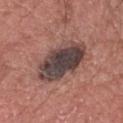Clinical impression: Recorded during total-body skin imaging; not selected for excision or biopsy. Background: Automated image analysis of the tile measured a lesion area of about 20 mm², an eccentricity of roughly 0.7, and two-axis asymmetry of about 0.15. The software also gave an average lesion color of about L≈41 a*≈16 b*≈17 (CIELAB). The analysis additionally found a border-irregularity rating of about 2/10 and peripheral color asymmetry of about 2. Measured at roughly 6 mm in maximum diameter. A roughly 15 mm field-of-view crop from a total-body skin photograph. On the head or neck. A male patient, aged approximately 75. The tile uses white-light illumination.